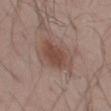Assessment:
This lesion was catalogued during total-body skin photography and was not selected for biopsy.
Image and clinical context:
This is a white-light tile. A roughly 15 mm field-of-view crop from a total-body skin photograph. An algorithmic analysis of the crop reported a mean CIELAB color near L≈46 a*≈19 b*≈24, about 9 CIELAB-L* units darker than the surrounding skin, and a lesion-to-skin contrast of about 7.5 (normalized; higher = more distinct). Approximately 5.5 mm at its widest. The subject is a male aged around 55. The lesion is located on the left thigh.